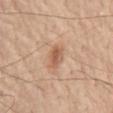Notes:
– workup · imaged on a skin check; not biopsied
– lighting · white-light illumination
– subject · male, aged 68 to 72
– lesion diameter · ~3 mm (longest diameter)
– acquisition · ~15 mm crop, total-body skin-cancer survey
– site · the front of the torso